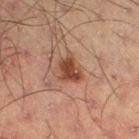notes = imaged on a skin check; not biopsied
image = ~15 mm crop, total-body skin-cancer survey
patient = male, aged 48 to 52
anatomic site = the right thigh